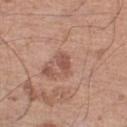– workup — catalogued during a skin exam; not biopsied
– TBP lesion metrics — a border-irregularity index near 2.5/10, internal color variation of about 2 on a 0–10 scale, and a peripheral color-asymmetry measure near 0.5
– image — 15 mm crop, total-body photography
– location — the left lower leg
– subject — male, aged around 70
– lesion diameter — about 3 mm
– tile lighting — white-light illumination